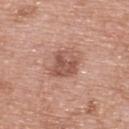{
  "biopsy_status": "not biopsied; imaged during a skin examination",
  "lesion_size": {
    "long_diameter_mm_approx": 3.0
  },
  "image": {
    "source": "total-body photography crop",
    "field_of_view_mm": 15
  },
  "lighting": "white-light",
  "site": "upper back",
  "patient": {
    "sex": "female",
    "age_approx": 60
  }
}